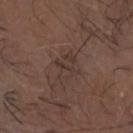Assessment: This lesion was catalogued during total-body skin photography and was not selected for biopsy. Acquisition and patient details: On the head or neck. A 15 mm close-up tile from a total-body photography series done for melanoma screening. The lesion-visualizer software estimated an outline eccentricity of about 0.75 (0 = round, 1 = elongated) and two-axis asymmetry of about 0.35. And it measured a mean CIELAB color near L≈34 a*≈14 b*≈20. The analysis additionally found a within-lesion color-variation index near 2.5/10 and peripheral color asymmetry of about 1. It also reported a classifier nevus-likeness of about 0/100 and a detector confidence of about 65 out of 100 that the crop contains a lesion. A male subject, in their mid- to late 60s. The tile uses cross-polarized illumination.